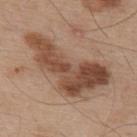notes: catalogued during a skin exam; not biopsied | image: ~15 mm tile from a whole-body skin photo | patient: male, aged around 55 | size: ~10 mm (longest diameter) | site: the back | automated metrics: a lesion area of about 29 mm², an outline eccentricity of about 0.9 (0 = round, 1 = elongated), and a symmetry-axis asymmetry near 0.55; a lesion color around L≈47 a*≈20 b*≈29 in CIELAB, a lesion–skin lightness drop of about 14, and a normalized border contrast of about 10; a color-variation rating of about 6.5/10 and a peripheral color-asymmetry measure near 2.5; an automated nevus-likeness rating near 65 out of 100 and a lesion-detection confidence of about 100/100.